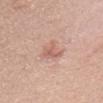Findings:
• workup · total-body-photography surveillance lesion; no biopsy
• automated lesion analysis · roughly 9 lightness units darker than nearby skin and a normalized border contrast of about 5.5; border irregularity of about 4.5 on a 0–10 scale, a color-variation rating of about 2.5/10, and peripheral color asymmetry of about 1; a classifier nevus-likeness of about 0/100 and lesion-presence confidence of about 100/100
• acquisition · total-body-photography crop, ~15 mm field of view
• size · ≈3.5 mm
• location · the front of the torso
• patient · male, approximately 55 years of age